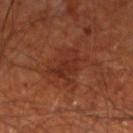Impression: The lesion was photographed on a routine skin check and not biopsied; there is no pathology result. Clinical summary: About 5 mm across. The patient is a male aged around 70. The lesion is located on the leg. Cropped from a whole-body photographic skin survey; the tile spans about 15 mm. An algorithmic analysis of the crop reported an area of roughly 10 mm², an outline eccentricity of about 0.8 (0 = round, 1 = elongated), and a shape-asymmetry score of about 0.4 (0 = symmetric). The software also gave a mean CIELAB color near L≈30 a*≈25 b*≈28, roughly 6 lightness units darker than nearby skin, and a normalized lesion–skin contrast near 6.5. It also reported a classifier nevus-likeness of about 0/100 and lesion-presence confidence of about 100/100. Captured under cross-polarized illumination.